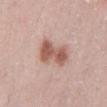Recorded during total-body skin imaging; not selected for excision or biopsy.
This is a white-light tile.
A 15 mm close-up extracted from a 3D total-body photography capture.
Measured at roughly 4.5 mm in maximum diameter.
Located on the abdomen.
A female patient in their 30s.
Automated tile analysis of the lesion measured an average lesion color of about L≈57 a*≈22 b*≈26 (CIELAB), a lesion–skin lightness drop of about 13, and a normalized border contrast of about 8.5. The software also gave a nevus-likeness score of about 95/100 and lesion-presence confidence of about 100/100.